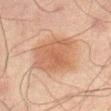biopsy status = catalogued during a skin exam; not biopsied | lesion diameter = about 7 mm | subject = male, aged approximately 65 | body site = the lower back | image = ~15 mm tile from a whole-body skin photo.